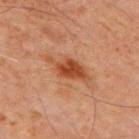No biopsy was performed on this lesion — it was imaged during a full skin examination and was not determined to be concerning.
An algorithmic analysis of the crop reported an average lesion color of about L≈40 a*≈23 b*≈32 (CIELAB) and roughly 10 lightness units darker than nearby skin. It also reported internal color variation of about 3 on a 0–10 scale and radial color variation of about 1. And it measured a nevus-likeness score of about 80/100 and a lesion-detection confidence of about 100/100.
The lesion is on the chest.
The lesion's longest dimension is about 5.5 mm.
A male patient, about 70 years old.
A close-up tile cropped from a whole-body skin photograph, about 15 mm across.